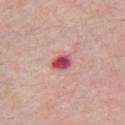workup = imaged on a skin check; not biopsied
image source = total-body-photography crop, ~15 mm field of view
subject = female, in their 70s
body site = the chest